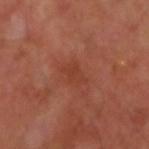Clinical impression:
Imaged during a routine full-body skin examination; the lesion was not biopsied and no histopathology is available.
Context:
The lesion's longest dimension is about 3 mm. A 15 mm close-up extracted from a 3D total-body photography capture. This is a cross-polarized tile. On the left arm. The patient is a male aged 48 to 52.